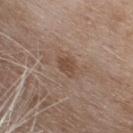Q: Is there a histopathology result?
A: imaged on a skin check; not biopsied
Q: Patient demographics?
A: female, aged 73 to 77
Q: What is the imaging modality?
A: total-body-photography crop, ~15 mm field of view
Q: Lesion location?
A: the upper back